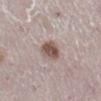The lesion was photographed on a routine skin check and not biopsied; there is no pathology result.